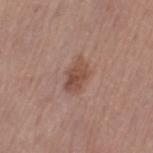| key | value |
|---|---|
| workup | no biopsy performed (imaged during a skin exam) |
| lesion size | about 4 mm |
| image | ~15 mm tile from a whole-body skin photo |
| TBP lesion metrics | an area of roughly 7 mm² and two-axis asymmetry of about 0.25; a nevus-likeness score of about 60/100 and a detector confidence of about 100 out of 100 that the crop contains a lesion |
| subject | female, aged approximately 65 |
| tile lighting | white-light illumination |
| location | the left thigh |